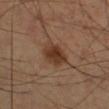Acquisition and patient details:
Automated tile analysis of the lesion measured a nevus-likeness score of about 95/100 and a lesion-detection confidence of about 100/100. A lesion tile, about 15 mm wide, cut from a 3D total-body photograph. The recorded lesion diameter is about 3.5 mm. Imaged with cross-polarized lighting. The lesion is located on the right lower leg. The subject is a male aged 53 to 57.